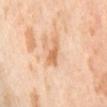Clinical impression:
Captured during whole-body skin photography for melanoma surveillance; the lesion was not biopsied.
Context:
A female patient in their mid- to late 50s. The lesion is located on the back. This is a cross-polarized tile. Measured at roughly 3 mm in maximum diameter. A lesion tile, about 15 mm wide, cut from a 3D total-body photograph.